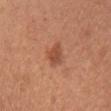No biopsy was performed on this lesion — it was imaged during a full skin examination and was not determined to be concerning. A female subject, aged approximately 25. The lesion-visualizer software estimated a lesion area of about 5 mm². This image is a 15 mm lesion crop taken from a total-body photograph. Imaged with white-light lighting. From the chest.